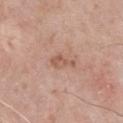No biopsy was performed on this lesion — it was imaged during a full skin examination and was not determined to be concerning. A male subject aged approximately 60. The tile uses white-light illumination. A 15 mm close-up extracted from a 3D total-body photography capture. From the chest. The lesion's longest dimension is about 3 mm. The total-body-photography lesion software estimated a footprint of about 3.5 mm², an eccentricity of roughly 0.9, and two-axis asymmetry of about 0.45. The software also gave about 9 CIELAB-L* units darker than the surrounding skin and a normalized border contrast of about 6.5.